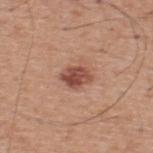Recorded during total-body skin imaging; not selected for excision or biopsy.
The lesion is located on the upper back.
A male patient in their 60s.
A 15 mm close-up extracted from a 3D total-body photography capture.